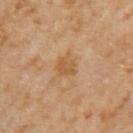<tbp_lesion>
<biopsy_status>not biopsied; imaged during a skin examination</biopsy_status>
<lighting>cross-polarized</lighting>
<patient>
  <sex>female</sex>
  <age_approx>60</age_approx>
</patient>
<image>
  <source>total-body photography crop</source>
  <field_of_view_mm>15</field_of_view_mm>
</image>
<site>left upper arm</site>
<automated_metrics>
  <eccentricity>0.25</eccentricity>
  <shape_asymmetry>0.25</shape_asymmetry>
  <border_irregularity_0_10>2.5</border_irregularity_0_10>
  <peripheral_color_asymmetry>0.5</peripheral_color_asymmetry>
  <nevus_likeness_0_100>0</nevus_likeness_0_100>
  <lesion_detection_confidence_0_100>100</lesion_detection_confidence_0_100>
</automated_metrics>
<lesion_size>
  <long_diameter_mm_approx>2.5</long_diameter_mm_approx>
</lesion_size>
</tbp_lesion>